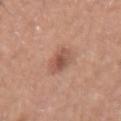Acquisition and patient details: The patient is a female aged approximately 40. The lesion is on the right forearm. This image is a 15 mm lesion crop taken from a total-body photograph.